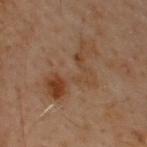Q: Was a biopsy performed?
A: catalogued during a skin exam; not biopsied
Q: What is the anatomic site?
A: the upper back
Q: What are the patient's age and sex?
A: male, aged approximately 50
Q: Lesion size?
A: about 8 mm
Q: What kind of image is this?
A: 15 mm crop, total-body photography
Q: How was the tile lit?
A: cross-polarized